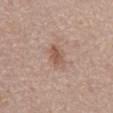Imaged during a routine full-body skin examination; the lesion was not biopsied and no histopathology is available.
This image is a 15 mm lesion crop taken from a total-body photograph.
The patient is a male aged 68–72.
On the mid back.